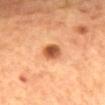The lesion was photographed on a routine skin check and not biopsied; there is no pathology result. The recorded lesion diameter is about 2.5 mm. A lesion tile, about 15 mm wide, cut from a 3D total-body photograph. The lesion is on the mid back. The subject is a female aged 58–62. The tile uses cross-polarized illumination.